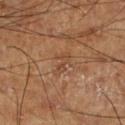Recorded during total-body skin imaging; not selected for excision or biopsy. The tile uses cross-polarized illumination. Located on the right lower leg. The lesion-visualizer software estimated an area of roughly 3.5 mm², an eccentricity of roughly 0.85, and two-axis asymmetry of about 0.5. The analysis additionally found a lesion color around L≈45 a*≈21 b*≈32 in CIELAB and a lesion-to-skin contrast of about 5 (normalized; higher = more distinct). The analysis additionally found a classifier nevus-likeness of about 0/100 and lesion-presence confidence of about 100/100. Cropped from a total-body skin-imaging series; the visible field is about 15 mm. A male patient, aged around 70.